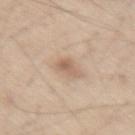Findings:
- notes · no biopsy performed (imaged during a skin exam)
- illumination · white-light
- image source · total-body-photography crop, ~15 mm field of view
- site · the right thigh
- subject · male, aged around 60